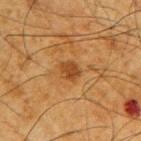Assessment:
Recorded during total-body skin imaging; not selected for excision or biopsy.
Background:
A 15 mm close-up tile from a total-body photography series done for melanoma screening. The total-body-photography lesion software estimated a lesion color around L≈37 a*≈21 b*≈35 in CIELAB, about 9 CIELAB-L* units darker than the surrounding skin, and a lesion-to-skin contrast of about 7.5 (normalized; higher = more distinct). The analysis additionally found a nevus-likeness score of about 45/100 and a detector confidence of about 100 out of 100 that the crop contains a lesion. Approximately 2.5 mm at its widest. A male patient, approximately 65 years of age. The lesion is located on the right upper arm. Imaged with cross-polarized lighting.